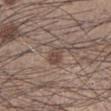Clinical impression:
This lesion was catalogued during total-body skin photography and was not selected for biopsy.
Background:
The patient is a male aged around 35. Imaged with white-light lighting. A roughly 15 mm field-of-view crop from a total-body skin photograph. The lesion's longest dimension is about 3 mm. On the left lower leg. An algorithmic analysis of the crop reported an average lesion color of about L≈46 a*≈15 b*≈23 (CIELAB) and a normalized lesion–skin contrast near 6.5. The software also gave a border-irregularity index near 2/10, a color-variation rating of about 3/10, and radial color variation of about 1.